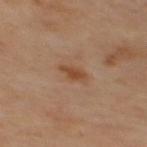{"biopsy_status": "not biopsied; imaged during a skin examination", "lesion_size": {"long_diameter_mm_approx": 3.0}, "lighting": "cross-polarized", "image": {"source": "total-body photography crop", "field_of_view_mm": 15}, "automated_metrics": {"area_mm2_approx": 3.5, "shape_asymmetry": 0.35, "cielab_L": 47, "cielab_a": 22, "cielab_b": 34, "vs_skin_contrast_norm": 7.5, "border_irregularity_0_10": 3.5, "color_variation_0_10": 1.5, "peripheral_color_asymmetry": 0.5}, "site": "back"}